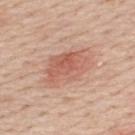This lesion was catalogued during total-body skin photography and was not selected for biopsy. An algorithmic analysis of the crop reported an eccentricity of roughly 0.8 and two-axis asymmetry of about 0.2. And it measured a mean CIELAB color near L≈60 a*≈24 b*≈29, about 9 CIELAB-L* units darker than the surrounding skin, and a lesion-to-skin contrast of about 6 (normalized; higher = more distinct). The software also gave a border-irregularity index near 2.5/10 and internal color variation of about 5 on a 0–10 scale. It also reported a nevus-likeness score of about 95/100 and a lesion-detection confidence of about 100/100. Captured under white-light illumination. Located on the upper back. A male patient aged 58–62. Approximately 6.5 mm at its widest. A lesion tile, about 15 mm wide, cut from a 3D total-body photograph.